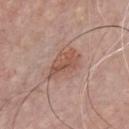  biopsy_status: not biopsied; imaged during a skin examination
  lighting: white-light
  site: chest
  patient:
    sex: male
    age_approx: 65
  image:
    source: total-body photography crop
    field_of_view_mm: 15
  lesion_size:
    long_diameter_mm_approx: 4.5